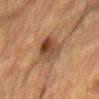Impression:
Part of a total-body skin-imaging series; this lesion was reviewed on a skin check and was not flagged for biopsy.
Clinical summary:
Approximately 4 mm at its widest. A 15 mm crop from a total-body photograph taken for skin-cancer surveillance. A male patient roughly 85 years of age or older. Captured under cross-polarized illumination. On the mid back.